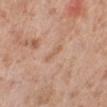site = the left lower leg
acquisition = 15 mm crop, total-body photography
subject = male, approximately 80 years of age